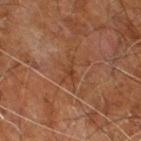Part of a total-body skin-imaging series; this lesion was reviewed on a skin check and was not flagged for biopsy. Captured under cross-polarized illumination. The lesion is located on the leg. Measured at roughly 3 mm in maximum diameter. A male patient in their 60s. A roughly 15 mm field-of-view crop from a total-body skin photograph.